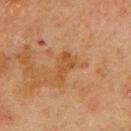The lesion was photographed on a routine skin check and not biopsied; there is no pathology result. The patient is a male aged 68 to 72. The total-body-photography lesion software estimated a lesion color around L≈41 a*≈21 b*≈34 in CIELAB, roughly 6 lightness units darker than nearby skin, and a normalized lesion–skin contrast near 6. Located on the mid back. A 15 mm close-up tile from a total-body photography series done for melanoma screening. The lesion's longest dimension is about 3.5 mm.